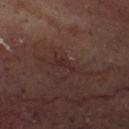<lesion>
<biopsy_status>not biopsied; imaged during a skin examination</biopsy_status>
<site>head or neck</site>
<image>
  <source>total-body photography crop</source>
  <field_of_view_mm>15</field_of_view_mm>
</image>
<patient>
  <sex>male</sex>
  <age_approx>65</age_approx>
</patient>
<lighting>cross-polarized</lighting>
</lesion>